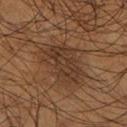Impression: This lesion was catalogued during total-body skin photography and was not selected for biopsy. Image and clinical context: About 5.5 mm across. The tile uses cross-polarized illumination. A region of skin cropped from a whole-body photographic capture, roughly 15 mm wide. A male subject about 55 years old. Located on the leg.